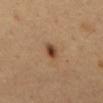Clinical impression: This lesion was catalogued during total-body skin photography and was not selected for biopsy. Acquisition and patient details: A 15 mm crop from a total-body photograph taken for skin-cancer surveillance. From the abdomen. The tile uses cross-polarized illumination. A male subject aged approximately 65. The recorded lesion diameter is about 2.5 mm.